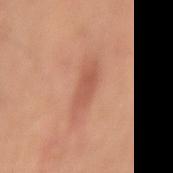Q: Is there a histopathology result?
A: total-body-photography surveillance lesion; no biopsy